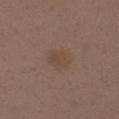{"biopsy_status": "not biopsied; imaged during a skin examination", "image": {"source": "total-body photography crop", "field_of_view_mm": 15}, "lesion_size": {"long_diameter_mm_approx": 3.0}, "patient": {"sex": "male", "age_approx": 40}, "automated_metrics": {"cielab_L": 44, "cielab_a": 15, "cielab_b": 26, "vs_skin_darker_L": 5.0, "vs_skin_contrast_norm": 5.0, "color_variation_0_10": 1.5, "peripheral_color_asymmetry": 0.5, "nevus_likeness_0_100": 5}, "site": "left upper arm", "lighting": "white-light"}